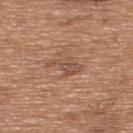Assessment:
Imaged during a routine full-body skin examination; the lesion was not biopsied and no histopathology is available.
Clinical summary:
A male subject, about 65 years old. An algorithmic analysis of the crop reported an eccentricity of roughly 0.85. It also reported a mean CIELAB color near L≈51 a*≈21 b*≈29 and a normalized border contrast of about 6. The lesion is on the upper back. Captured under white-light illumination. A 15 mm close-up tile from a total-body photography series done for melanoma screening.